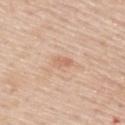follow-up — no biopsy performed (imaged during a skin exam).